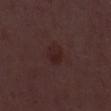biopsy status = imaged on a skin check; not biopsied
patient = male, about 50 years old
lesion diameter = ~3 mm (longest diameter)
TBP lesion metrics = an eccentricity of roughly 0.75 and a symmetry-axis asymmetry near 0.2; a detector confidence of about 100 out of 100 that the crop contains a lesion
image source = 15 mm crop, total-body photography